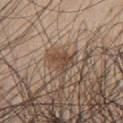notes: imaged on a skin check; not biopsied | subject: male, in their 60s | diameter: about 3.5 mm | TBP lesion metrics: a lesion area of about 6 mm² and two-axis asymmetry of about 0.3; about 9 CIELAB-L* units darker than the surrounding skin and a normalized lesion–skin contrast near 7.5; border irregularity of about 3.5 on a 0–10 scale and peripheral color asymmetry of about 1.5; lesion-presence confidence of about 75/100 | imaging modality: ~15 mm tile from a whole-body skin photo | location: the chest | lighting: white-light illumination.